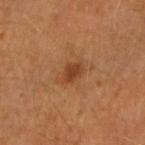Image and clinical context:
A region of skin cropped from a whole-body photographic capture, roughly 15 mm wide. A female subject, approximately 50 years of age. The lesion is located on the right forearm. Measured at roughly 3 mm in maximum diameter. Imaged with cross-polarized lighting.